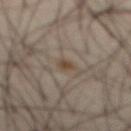  biopsy_status: not biopsied; imaged during a skin examination
  lesion_size:
    long_diameter_mm_approx: 1.5
  site: abdomen
  lighting: cross-polarized
  image:
    source: total-body photography crop
    field_of_view_mm: 15
  patient:
    sex: male
    age_approx: 45